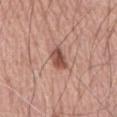- follow-up: total-body-photography surveillance lesion; no biopsy
- body site: the front of the torso
- image-analysis metrics: a mean CIELAB color near L≈51 a*≈23 b*≈27, about 13 CIELAB-L* units darker than the surrounding skin, and a lesion-to-skin contrast of about 9 (normalized; higher = more distinct); border irregularity of about 2.5 on a 0–10 scale and a within-lesion color-variation index near 4/10
- lesion diameter: ~3.5 mm (longest diameter)
- image: ~15 mm tile from a whole-body skin photo
- subject: male, roughly 65 years of age